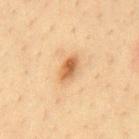Captured during whole-body skin photography for melanoma surveillance; the lesion was not biopsied. Cropped from a total-body skin-imaging series; the visible field is about 15 mm. Imaged with cross-polarized lighting. Located on the mid back. The lesion-visualizer software estimated a symmetry-axis asymmetry near 0.2. It also reported a lesion color around L≈58 a*≈21 b*≈39 in CIELAB, about 13 CIELAB-L* units darker than the surrounding skin, and a normalized lesion–skin contrast near 8.5. And it measured a within-lesion color-variation index near 4/10 and peripheral color asymmetry of about 1. The analysis additionally found a nevus-likeness score of about 95/100 and a lesion-detection confidence of about 100/100. Longest diameter approximately 3.5 mm. A male subject, aged approximately 35.